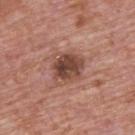A male patient aged 73 to 77. The lesion is on the upper back. Measured at roughly 4 mm in maximum diameter. A 15 mm close-up tile from a total-body photography series done for melanoma screening.